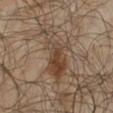notes=catalogued during a skin exam; not biopsied
patient=male, aged 63 to 67
image=~15 mm crop, total-body skin-cancer survey
lesion diameter=about 7 mm
tile lighting=cross-polarized illumination
TBP lesion metrics=a nevus-likeness score of about 30/100 and lesion-presence confidence of about 100/100
site=the left lower leg